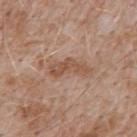Q: Was this lesion biopsied?
A: no biopsy performed (imaged during a skin exam)
Q: What is the lesion's diameter?
A: about 4.5 mm
Q: What is the anatomic site?
A: the upper back
Q: Who is the patient?
A: male, in their 50s
Q: What kind of image is this?
A: 15 mm crop, total-body photography
Q: What lighting was used for the tile?
A: white-light
Q: Automated lesion metrics?
A: a lesion area of about 7 mm² and a shape eccentricity near 0.9; a nevus-likeness score of about 0/100 and a lesion-detection confidence of about 100/100